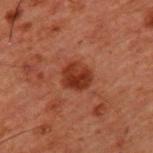<case>
  <patient>
    <sex>male</sex>
    <age_approx>60</age_approx>
  </patient>
  <automated_metrics>
    <nevus_likeness_0_100>90</nevus_likeness_0_100>
  </automated_metrics>
  <lesion_size>
    <long_diameter_mm_approx>3.0</long_diameter_mm_approx>
  </lesion_size>
  <lighting>cross-polarized</lighting>
  <image>
    <source>total-body photography crop</source>
    <field_of_view_mm>15</field_of_view_mm>
  </image>
  <site>upper back</site>
</case>